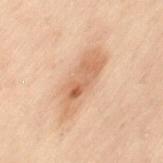{
  "biopsy_status": "not biopsied; imaged during a skin examination",
  "image": {
    "source": "total-body photography crop",
    "field_of_view_mm": 15
  },
  "site": "back",
  "patient": {
    "sex": "female",
    "age_approx": 55
  }
}